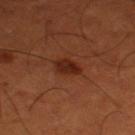workup = no biopsy performed (imaged during a skin exam) | lighting = cross-polarized illumination | image source = total-body-photography crop, ~15 mm field of view | size = ≈3 mm | patient = male, aged 48 to 52 | location = the leg | TBP lesion metrics = a mean CIELAB color near L≈26 a*≈25 b*≈29, roughly 9 lightness units darker than nearby skin, and a normalized lesion–skin contrast near 9; a classifier nevus-likeness of about 95/100 and lesion-presence confidence of about 100/100.